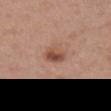Imaged during a routine full-body skin examination; the lesion was not biopsied and no histopathology is available. Approximately 2.5 mm at its widest. The subject is a female approximately 60 years of age. A 15 mm close-up extracted from a 3D total-body photography capture. The lesion is located on the chest.